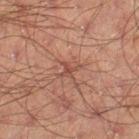<tbp_lesion>
<biopsy_status>not biopsied; imaged during a skin examination</biopsy_status>
<image>
  <source>total-body photography crop</source>
  <field_of_view_mm>15</field_of_view_mm>
</image>
<site>leg</site>
<patient>
  <sex>male</sex>
  <age_approx>50</age_approx>
</patient>
</tbp_lesion>